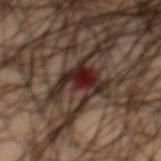Findings:
- notes — no biopsy performed (imaged during a skin exam)
- lesion diameter — about 5 mm
- image source — total-body-photography crop, ~15 mm field of view
- location — the mid back
- automated lesion analysis — a footprint of about 11 mm², an eccentricity of roughly 0.4, and two-axis asymmetry of about 0.6
- subject — male, aged 48 to 52